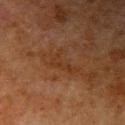{"biopsy_status": "not biopsied; imaged during a skin examination", "patient": {"sex": "male", "age_approx": 80}, "lighting": "cross-polarized", "image": {"source": "total-body photography crop", "field_of_view_mm": 15}, "site": "right upper arm"}